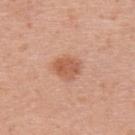Assessment: No biopsy was performed on this lesion — it was imaged during a full skin examination and was not determined to be concerning. Clinical summary: The lesion is located on the upper back. An algorithmic analysis of the crop reported an average lesion color of about L≈57 a*≈25 b*≈33 (CIELAB), about 10 CIELAB-L* units darker than the surrounding skin, and a normalized lesion–skin contrast near 7. The software also gave a border-irregularity rating of about 2/10 and a color-variation rating of about 2.5/10. The software also gave a nevus-likeness score of about 95/100 and lesion-presence confidence of about 100/100. A 15 mm close-up extracted from a 3D total-body photography capture. Captured under white-light illumination. The patient is a female aged approximately 30. Approximately 3 mm at its widest.